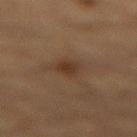This lesion was catalogued during total-body skin photography and was not selected for biopsy.
From the back.
Captured under cross-polarized illumination.
About 2.5 mm across.
The total-body-photography lesion software estimated a footprint of about 4 mm², a shape eccentricity near 0.65, and a symmetry-axis asymmetry near 0.2. It also reported border irregularity of about 2 on a 0–10 scale and a color-variation rating of about 1.5/10.
A 15 mm close-up extracted from a 3D total-body photography capture.
A male subject about 85 years old or older.